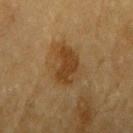This lesion was catalogued during total-body skin photography and was not selected for biopsy. On the arm. An algorithmic analysis of the crop reported a lesion area of about 13 mm² and an outline eccentricity of about 0.75 (0 = round, 1 = elongated). A male subject in their mid- to late 80s or older. Measured at roughly 5 mm in maximum diameter. A 15 mm crop from a total-body photograph taken for skin-cancer surveillance. The tile uses cross-polarized illumination.